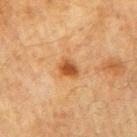Case summary:
– follow-up — no biopsy performed (imaged during a skin exam)
– location — the arm
– acquisition — total-body-photography crop, ~15 mm field of view
– lighting — cross-polarized illumination
– subject — male, aged 68–72